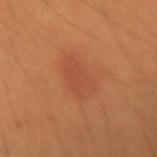Q: Was a biopsy performed?
A: no biopsy performed (imaged during a skin exam)